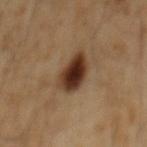No biopsy was performed on this lesion — it was imaged during a full skin examination and was not determined to be concerning.